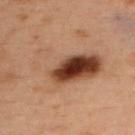A female patient, aged around 55.
A lesion tile, about 15 mm wide, cut from a 3D total-body photograph.
The total-body-photography lesion software estimated a lesion area of about 29 mm², an outline eccentricity of about 0.85 (0 = round, 1 = elongated), and a shape-asymmetry score of about 0.4 (0 = symmetric). The analysis additionally found a within-lesion color-variation index near 10/10 and peripheral color asymmetry of about 6.5. The analysis additionally found an automated nevus-likeness rating near 100 out of 100 and a lesion-detection confidence of about 100/100.
On the back.
Imaged with cross-polarized lighting.